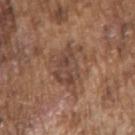Q: Is there a histopathology result?
A: total-body-photography surveillance lesion; no biopsy
Q: Patient demographics?
A: male, approximately 75 years of age
Q: How was this image acquired?
A: ~15 mm crop, total-body skin-cancer survey
Q: Where on the body is the lesion?
A: the right upper arm
Q: What lighting was used for the tile?
A: white-light illumination
Q: Lesion size?
A: ~6 mm (longest diameter)
Q: Automated lesion metrics?
A: an eccentricity of roughly 0.8